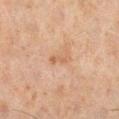location=the right lower leg
patient=male, approximately 45 years of age
illumination=cross-polarized
lesion diameter=≈2.5 mm
acquisition=~15 mm tile from a whole-body skin photo